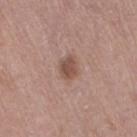Recorded during total-body skin imaging; not selected for excision or biopsy.
Imaged with white-light lighting.
Measured at roughly 2.5 mm in maximum diameter.
On the left thigh.
A female subject, in their mid- to late 60s.
Automated tile analysis of the lesion measured a lesion area of about 4.5 mm², an eccentricity of roughly 0.6, and a symmetry-axis asymmetry near 0.25. And it measured a nevus-likeness score of about 80/100 and a detector confidence of about 100 out of 100 that the crop contains a lesion.
This image is a 15 mm lesion crop taken from a total-body photograph.